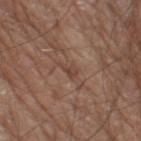biopsy_status: not biopsied; imaged during a skin examination
lighting: white-light
image:
  source: total-body photography crop
  field_of_view_mm: 15
site: right thigh
patient:
  sex: male
  age_approx: 80
automated_metrics:
  cielab_L: 43
  cielab_a: 19
  cielab_b: 26
  vs_skin_darker_L: 7.0
  vs_skin_contrast_norm: 5.5
  border_irregularity_0_10: 5.0
  color_variation_0_10: 1.0
  peripheral_color_asymmetry: 0.5
  nevus_likeness_0_100: 0
  lesion_detection_confidence_0_100: 65